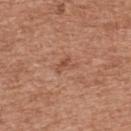This lesion was catalogued during total-body skin photography and was not selected for biopsy. The tile uses white-light illumination. A female patient, aged 58 to 62. The lesion is on the upper back. About 3 mm across. Automated image analysis of the tile measured a within-lesion color-variation index near 2/10 and a peripheral color-asymmetry measure near 1. A 15 mm close-up tile from a total-body photography series done for melanoma screening.